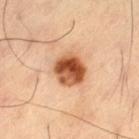No biopsy was performed on this lesion — it was imaged during a full skin examination and was not determined to be concerning. Located on the left thigh. Approximately 4 mm at its widest. This is a cross-polarized tile. A male subject approximately 60 years of age. A 15 mm crop from a total-body photograph taken for skin-cancer surveillance.